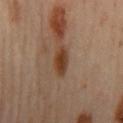No biopsy was performed on this lesion — it was imaged during a full skin examination and was not determined to be concerning. The lesion is on the mid back. The patient is a male approximately 65 years of age. A lesion tile, about 15 mm wide, cut from a 3D total-body photograph.